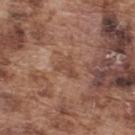Case summary:
– notes — imaged on a skin check; not biopsied
– anatomic site — the upper back
– automated metrics — an area of roughly 4 mm² and an eccentricity of roughly 0.65; a mean CIELAB color near L≈47 a*≈20 b*≈28, roughly 8 lightness units darker than nearby skin, and a normalized border contrast of about 6; border irregularity of about 6.5 on a 0–10 scale, a color-variation rating of about 1/10, and a peripheral color-asymmetry measure near 0.5; a nevus-likeness score of about 0/100 and lesion-presence confidence of about 90/100
– tile lighting — white-light illumination
– image — 15 mm crop, total-body photography
– subject — male, in their mid-70s